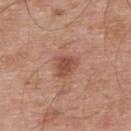follow-up: total-body-photography surveillance lesion; no biopsy
patient: male, aged 53–57
site: the upper back
image: total-body-photography crop, ~15 mm field of view